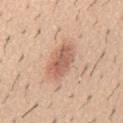Recorded during total-body skin imaging; not selected for excision or biopsy.
A male patient, aged approximately 60.
The tile uses white-light illumination.
A region of skin cropped from a whole-body photographic capture, roughly 15 mm wide.
The lesion-visualizer software estimated a mean CIELAB color near L≈61 a*≈22 b*≈30, roughly 11 lightness units darker than nearby skin, and a normalized lesion–skin contrast near 7. It also reported a detector confidence of about 100 out of 100 that the crop contains a lesion.
The lesion is on the mid back.